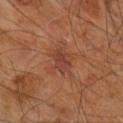Q: Was a biopsy performed?
A: total-body-photography surveillance lesion; no biopsy
Q: Who is the patient?
A: male, aged 58–62
Q: Lesion location?
A: the right leg
Q: How was this image acquired?
A: ~15 mm tile from a whole-body skin photo